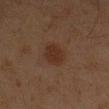Assessment: Recorded during total-body skin imaging; not selected for excision or biopsy. Image and clinical context: This image is a 15 mm lesion crop taken from a total-body photograph. Measured at roughly 3.5 mm in maximum diameter. Automated tile analysis of the lesion measured a footprint of about 7 mm² and a symmetry-axis asymmetry near 0.15. It also reported a nevus-likeness score of about 75/100 and a detector confidence of about 100 out of 100 that the crop contains a lesion. The subject is a male aged approximately 45. The tile uses cross-polarized illumination. From the left forearm.